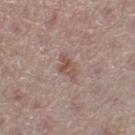This lesion was catalogued during total-body skin photography and was not selected for biopsy.
A female subject, aged approximately 50.
Automated image analysis of the tile measured a border-irregularity rating of about 4.5/10, a within-lesion color-variation index near 2.5/10, and a peripheral color-asymmetry measure near 0.5.
The lesion's longest dimension is about 3 mm.
The lesion is located on the leg.
A 15 mm crop from a total-body photograph taken for skin-cancer surveillance.
This is a white-light tile.